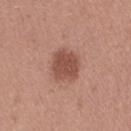* follow-up: no biopsy performed (imaged during a skin exam)
* image source: ~15 mm crop, total-body skin-cancer survey
* body site: the right thigh
* TBP lesion metrics: a footprint of about 9.5 mm², a shape eccentricity near 0.6, and a shape-asymmetry score of about 0.15 (0 = symmetric); a mean CIELAB color near L≈49 a*≈24 b*≈27 and roughly 12 lightness units darker than nearby skin; a border-irregularity index near 1.5/10 and a peripheral color-asymmetry measure near 0.5
* diameter: ≈4 mm
* patient: female, in their 40s
* lighting: white-light